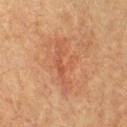Assessment:
Imaged during a routine full-body skin examination; the lesion was not biopsied and no histopathology is available.
Context:
Measured at roughly 6.5 mm in maximum diameter. On the front of the torso. A close-up tile cropped from a whole-body skin photograph, about 15 mm across. Automated image analysis of the tile measured an area of roughly 9.5 mm², an outline eccentricity of about 0.95 (0 = round, 1 = elongated), and a symmetry-axis asymmetry near 0.45. The software also gave a mean CIELAB color near L≈50 a*≈24 b*≈33, a lesion–skin lightness drop of about 6, and a normalized border contrast of about 5. The analysis additionally found a border-irregularity rating of about 8.5/10 and radial color variation of about 0. A male patient, aged around 65.